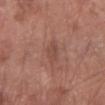patient: female, aged 68–72 | image: ~15 mm tile from a whole-body skin photo | anatomic site: the left forearm.